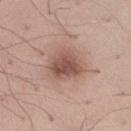Assessment: Captured during whole-body skin photography for melanoma surveillance; the lesion was not biopsied. Image and clinical context: Captured under white-light illumination. The total-body-photography lesion software estimated a footprint of about 11 mm², a shape eccentricity near 0.55, and a symmetry-axis asymmetry near 0.25. It also reported a lesion color around L≈52 a*≈20 b*≈25 in CIELAB, a lesion–skin lightness drop of about 12, and a lesion-to-skin contrast of about 8.5 (normalized; higher = more distinct). Measured at roughly 4 mm in maximum diameter. A lesion tile, about 15 mm wide, cut from a 3D total-body photograph. The lesion is located on the right thigh. A male subject, aged approximately 30.